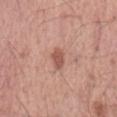<tbp_lesion>
  <biopsy_status>not biopsied; imaged during a skin examination</biopsy_status>
  <patient>
    <sex>male</sex>
    <age_approx>55</age_approx>
  </patient>
  <site>mid back</site>
  <automated_metrics>
    <area_mm2_approx>3.5</area_mm2_approx>
    <shape_asymmetry>0.3</shape_asymmetry>
    <border_irregularity_0_10>2.5</border_irregularity_0_10>
    <color_variation_0_10>2.0</color_variation_0_10>
    <nevus_likeness_0_100>75</nevus_likeness_0_100>
  </automated_metrics>
  <lighting>white-light</lighting>
  <lesion_size>
    <long_diameter_mm_approx>2.5</long_diameter_mm_approx>
  </lesion_size>
  <image>
    <source>total-body photography crop</source>
    <field_of_view_mm>15</field_of_view_mm>
  </image>
</tbp_lesion>